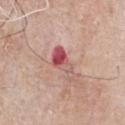Case summary:
– notes — total-body-photography surveillance lesion; no biopsy
– image source — 15 mm crop, total-body photography
– lighting — white-light illumination
– image-analysis metrics — an area of roughly 7 mm², a shape eccentricity near 0.9, and a symmetry-axis asymmetry near 0.45; an average lesion color of about L≈55 a*≈29 b*≈22 (CIELAB), roughly 12 lightness units darker than nearby skin, and a lesion-to-skin contrast of about 8.5 (normalized; higher = more distinct); a border-irregularity index near 4.5/10 and peripheral color asymmetry of about 5.5
– subject — male, aged approximately 65
– location — the chest
– lesion diameter — ~4.5 mm (longest diameter)